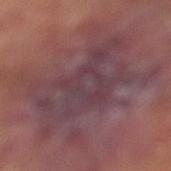Captured during whole-body skin photography for melanoma surveillance; the lesion was not biopsied.
The recorded lesion diameter is about 9.5 mm.
A male patient roughly 65 years of age.
Cropped from a total-body skin-imaging series; the visible field is about 15 mm.
The lesion is located on the left lower leg.
This is a cross-polarized tile.